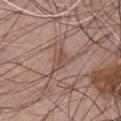<case>
<biopsy_status>not biopsied; imaged during a skin examination</biopsy_status>
<image>
  <source>total-body photography crop</source>
  <field_of_view_mm>15</field_of_view_mm>
</image>
<patient>
  <sex>male</sex>
  <age_approx>50</age_approx>
</patient>
<lesion_size>
  <long_diameter_mm_approx>3.0</long_diameter_mm_approx>
</lesion_size>
<lighting>white-light</lighting>
<site>front of the torso</site>
</case>